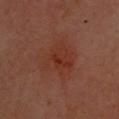notes: no biopsy performed (imaged during a skin exam) | patient: male, in their mid-60s | lesion diameter: ≈5 mm | image source: ~15 mm tile from a whole-body skin photo | automated metrics: a lesion area of about 14 mm², an eccentricity of roughly 0.65, and two-axis asymmetry of about 0.3; a mean CIELAB color near L≈32 a*≈24 b*≈27, a lesion–skin lightness drop of about 5, and a lesion-to-skin contrast of about 5.5 (normalized; higher = more distinct) | location: the head or neck.